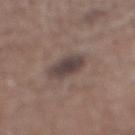Impression:
No biopsy was performed on this lesion — it was imaged during a full skin examination and was not determined to be concerning.
Background:
Located on the leg. A region of skin cropped from a whole-body photographic capture, roughly 15 mm wide. A male subject about 65 years old.